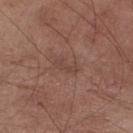Clinical summary: From the right lower leg. The patient is a male in their mid-50s. This image is a 15 mm lesion crop taken from a total-body photograph. The tile uses white-light illumination. Measured at roughly 3 mm in maximum diameter. An algorithmic analysis of the crop reported a footprint of about 3.5 mm² and an eccentricity of roughly 0.9. And it measured a mean CIELAB color near L≈43 a*≈19 b*≈23, roughly 6 lightness units darker than nearby skin, and a lesion-to-skin contrast of about 5 (normalized; higher = more distinct). The software also gave a border-irregularity rating of about 4/10, a color-variation rating of about 0.5/10, and a peripheral color-asymmetry measure near 0. The software also gave a classifier nevus-likeness of about 0/100 and a lesion-detection confidence of about 100/100.